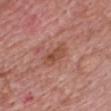Context:
Automated image analysis of the tile measured an area of roughly 6.5 mm², an outline eccentricity of about 0.9 (0 = round, 1 = elongated), and a symmetry-axis asymmetry near 0.3. And it measured a lesion color around L≈50 a*≈25 b*≈29 in CIELAB and a lesion–skin lightness drop of about 8. It also reported border irregularity of about 3 on a 0–10 scale, internal color variation of about 4 on a 0–10 scale, and a peripheral color-asymmetry measure near 1.5. The analysis additionally found an automated nevus-likeness rating near 5 out of 100 and a lesion-detection confidence of about 100/100. A region of skin cropped from a whole-body photographic capture, roughly 15 mm wide. Located on the front of the torso. Longest diameter approximately 4 mm. A male subject aged 58–62.